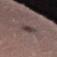Impression:
Recorded during total-body skin imaging; not selected for excision or biopsy.
Acquisition and patient details:
The lesion's longest dimension is about 4.5 mm. Located on the right thigh. The patient is a female approximately 40 years of age. Captured under white-light illumination. A region of skin cropped from a whole-body photographic capture, roughly 15 mm wide.